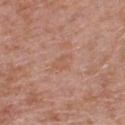The lesion was photographed on a routine skin check and not biopsied; there is no pathology result. The lesion is located on the right lower leg. A female patient in their 40s. Automated tile analysis of the lesion measured a lesion color around L≈56 a*≈22 b*≈31 in CIELAB, about 5 CIELAB-L* units darker than the surrounding skin, and a normalized border contrast of about 4.5. It also reported a color-variation rating of about 1/10. A close-up tile cropped from a whole-body skin photograph, about 15 mm across. The recorded lesion diameter is about 2.5 mm. This is a white-light tile.